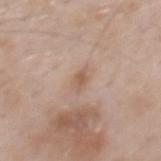<tbp_lesion>
  <biopsy_status>not biopsied; imaged during a skin examination</biopsy_status>
  <lesion_size>
    <long_diameter_mm_approx>2.5</long_diameter_mm_approx>
  </lesion_size>
  <automated_metrics>
    <area_mm2_approx>3.0</area_mm2_approx>
    <eccentricity>0.8</eccentricity>
    <shape_asymmetry>0.4</shape_asymmetry>
    <cielab_L>58</cielab_L>
    <cielab_a>17</cielab_a>
    <cielab_b>28</cielab_b>
    <vs_skin_darker_L>8.0</vs_skin_darker_L>
    <vs_skin_contrast_norm>6.0</vs_skin_contrast_norm>
    <nevus_likeness_0_100>0</nevus_likeness_0_100>
    <lesion_detection_confidence_0_100>100</lesion_detection_confidence_0_100>
  </automated_metrics>
  <patient>
    <sex>male</sex>
    <age_approx>60</age_approx>
  </patient>
  <lighting>white-light</lighting>
  <site>mid back</site>
  <image>
    <source>total-body photography crop</source>
    <field_of_view_mm>15</field_of_view_mm>
  </image>
</tbp_lesion>